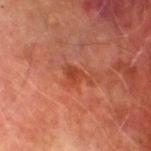Recorded during total-body skin imaging; not selected for excision or biopsy. Automated image analysis of the tile measured a lesion area of about 4 mm², an outline eccentricity of about 0.8 (0 = round, 1 = elongated), and a shape-asymmetry score of about 0.4 (0 = symmetric). A 15 mm close-up extracted from a 3D total-body photography capture. The recorded lesion diameter is about 3.5 mm. A male subject, roughly 75 years of age. The lesion is located on the leg. Imaged with cross-polarized lighting.